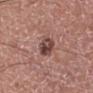No biopsy was performed on this lesion — it was imaged during a full skin examination and was not determined to be concerning. Located on the left lower leg. The subject is a male in their 60s. This image is a 15 mm lesion crop taken from a total-body photograph. This is a white-light tile. The lesion's longest dimension is about 3 mm. Automated tile analysis of the lesion measured a shape eccentricity near 0.6 and two-axis asymmetry of about 0.15. The software also gave an average lesion color of about L≈44 a*≈21 b*≈22 (CIELAB), roughly 13 lightness units darker than nearby skin, and a normalized lesion–skin contrast near 9.5.